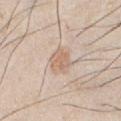<record>
  <biopsy_status>not biopsied; imaged during a skin examination</biopsy_status>
  <patient>
    <sex>male</sex>
    <age_approx>45</age_approx>
  </patient>
  <image>
    <source>total-body photography crop</source>
    <field_of_view_mm>15</field_of_view_mm>
  </image>
  <automated_metrics>
    <cielab_L>65</cielab_L>
    <cielab_a>17</cielab_a>
    <cielab_b>30</cielab_b>
    <vs_skin_darker_L>8.0</vs_skin_darker_L>
    <vs_skin_contrast_norm>6.0</vs_skin_contrast_norm>
    <color_variation_0_10>2.0</color_variation_0_10>
    <peripheral_color_asymmetry>0.5</peripheral_color_asymmetry>
  </automated_metrics>
  <site>front of the torso</site>
</record>